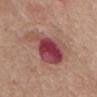Q: Was a biopsy performed?
A: no biopsy performed (imaged during a skin exam)
Q: Lesion location?
A: the abdomen
Q: Illumination type?
A: white-light illumination
Q: What kind of image is this?
A: ~15 mm crop, total-body skin-cancer survey
Q: How large is the lesion?
A: about 6 mm
Q: Patient demographics?
A: male, aged around 80